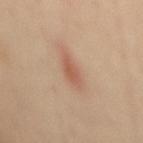Q: What is the lesion's diameter?
A: ≈3.5 mm
Q: What did automated image analysis measure?
A: a mean CIELAB color near L≈57 a*≈22 b*≈31, about 9 CIELAB-L* units darker than the surrounding skin, and a normalized border contrast of about 6.5; internal color variation of about 2.5 on a 0–10 scale and a peripheral color-asymmetry measure near 1; a nevus-likeness score of about 85/100 and a detector confidence of about 100 out of 100 that the crop contains a lesion
Q: What are the patient's age and sex?
A: female, roughly 40 years of age
Q: What is the imaging modality?
A: ~15 mm crop, total-body skin-cancer survey
Q: What lighting was used for the tile?
A: cross-polarized
Q: Lesion location?
A: the mid back